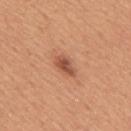Q: Was this lesion biopsied?
A: total-body-photography surveillance lesion; no biopsy